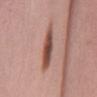Findings:
- acquisition — 15 mm crop, total-body photography
- lesion size — ≈5.5 mm
- patient — female, aged approximately 60
- site — the mid back